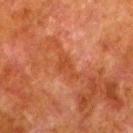The lesion was photographed on a routine skin check and not biopsied; there is no pathology result.
A region of skin cropped from a whole-body photographic capture, roughly 15 mm wide.
The lesion is located on the left lower leg.
This is a cross-polarized tile.
Approximately 3 mm at its widest.
A male subject aged approximately 80.